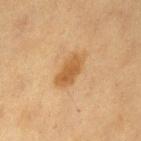<record>
<biopsy_status>not biopsied; imaged during a skin examination</biopsy_status>
<image>
  <source>total-body photography crop</source>
  <field_of_view_mm>15</field_of_view_mm>
</image>
<lighting>cross-polarized</lighting>
<automated_metrics>
  <border_irregularity_0_10>3.0</border_irregularity_0_10>
  <color_variation_0_10>3.0</color_variation_0_10>
</automated_metrics>
<site>leg</site>
<patient>
  <sex>female</sex>
  <age_approx>40</age_approx>
</patient>
<lesion_size>
  <long_diameter_mm_approx>5.0</long_diameter_mm_approx>
</lesion_size>
</record>